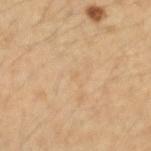biopsy status=catalogued during a skin exam; not biopsied | size=≈1 mm | subject=female, in their 30s | tile lighting=cross-polarized | site=the arm | imaging modality=~15 mm crop, total-body skin-cancer survey.